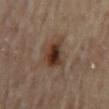biopsy status: imaged on a skin check; not biopsied
anatomic site: the mid back
imaging modality: total-body-photography crop, ~15 mm field of view
patient: female, roughly 60 years of age